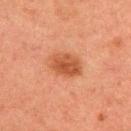The lesion was photographed on a routine skin check and not biopsied; there is no pathology result. A male patient in their 50s. A lesion tile, about 15 mm wide, cut from a 3D total-body photograph. The recorded lesion diameter is about 3.5 mm. This is a cross-polarized tile. On the upper back.